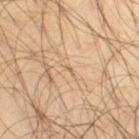The lesion is located on the right thigh.
A close-up tile cropped from a whole-body skin photograph, about 15 mm across.
Approximately 1 mm at its widest.
This is a cross-polarized tile.
The subject is a male aged 43–47.